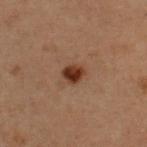* biopsy status — imaged on a skin check; not biopsied
* lesion diameter — about 2.5 mm
* site — the upper back
* illumination — cross-polarized
* TBP lesion metrics — a mean CIELAB color near L≈28 a*≈19 b*≈25; border irregularity of about 2 on a 0–10 scale and a peripheral color-asymmetry measure near 1; an automated nevus-likeness rating near 100 out of 100 and lesion-presence confidence of about 100/100
* image source — 15 mm crop, total-body photography
* subject — female, in their 30s